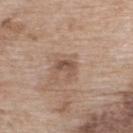Imaged during a routine full-body skin examination; the lesion was not biopsied and no histopathology is available. The tile uses white-light illumination. Automated tile analysis of the lesion measured roughly 10 lightness units darker than nearby skin and a lesion-to-skin contrast of about 7 (normalized; higher = more distinct). And it measured a classifier nevus-likeness of about 0/100 and lesion-presence confidence of about 100/100. Approximately 2.5 mm at its widest. From the upper back. This image is a 15 mm lesion crop taken from a total-body photograph. The subject is a female roughly 75 years of age.